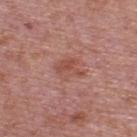follow-up: no biopsy performed (imaged during a skin exam)
diameter: ≈3.5 mm
body site: the upper back
automated metrics: a mean CIELAB color near L≈51 a*≈25 b*≈27; a nevus-likeness score of about 0/100 and a detector confidence of about 100 out of 100 that the crop contains a lesion
lighting: white-light
imaging modality: ~15 mm tile from a whole-body skin photo
patient: male, about 75 years old